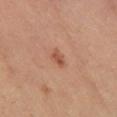Clinical impression: The lesion was tiled from a total-body skin photograph and was not biopsied. Image and clinical context: A female subject, aged approximately 30. Automated image analysis of the tile measured a shape eccentricity near 0.8 and two-axis asymmetry of about 0.3. It also reported a mean CIELAB color near L≈52 a*≈25 b*≈32, a lesion–skin lightness drop of about 9, and a normalized border contrast of about 7. Imaged with cross-polarized lighting. Cropped from a total-body skin-imaging series; the visible field is about 15 mm. Located on the left lower leg.